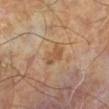  biopsy_status: not biopsied; imaged during a skin examination
  image:
    source: total-body photography crop
    field_of_view_mm: 15
  patient:
    sex: male
    age_approx: 65
  lighting: cross-polarized
  automated_metrics:
    border_irregularity_0_10: 3.5
    color_variation_0_10: 2.0
    peripheral_color_asymmetry: 0.5
    nevus_likeness_0_100: 0
    lesion_detection_confidence_0_100: 100
  lesion_size:
    long_diameter_mm_approx: 3.0